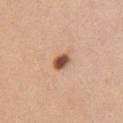  biopsy_status: not biopsied; imaged during a skin examination
  site: chest
  image:
    source: total-body photography crop
    field_of_view_mm: 15
  patient:
    sex: female
    age_approx: 40